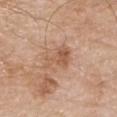This lesion was catalogued during total-body skin photography and was not selected for biopsy. The recorded lesion diameter is about 4.5 mm. On the arm. Cropped from a whole-body photographic skin survey; the tile spans about 15 mm. Imaged with white-light lighting. A male subject in their 80s. The lesion-visualizer software estimated a border-irregularity rating of about 5/10 and a peripheral color-asymmetry measure near 1.5. It also reported an automated nevus-likeness rating near 0 out of 100.